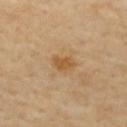Q: Was this lesion biopsied?
A: imaged on a skin check; not biopsied
Q: Lesion location?
A: the back
Q: Automated lesion metrics?
A: a lesion color around L≈56 a*≈19 b*≈40 in CIELAB, roughly 9 lightness units darker than nearby skin, and a normalized border contrast of about 7.5; a border-irregularity index near 3/10 and internal color variation of about 1.5 on a 0–10 scale; an automated nevus-likeness rating near 20 out of 100 and a detector confidence of about 100 out of 100 that the crop contains a lesion
Q: What is the lesion's diameter?
A: ~3 mm (longest diameter)
Q: What are the patient's age and sex?
A: female, in their 70s
Q: What is the imaging modality?
A: ~15 mm tile from a whole-body skin photo